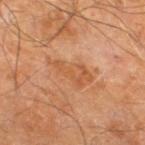Q: Was a biopsy performed?
A: no biopsy performed (imaged during a skin exam)
Q: What is the anatomic site?
A: the right leg
Q: What did automated image analysis measure?
A: an outline eccentricity of about 0.9 (0 = round, 1 = elongated) and a shape-asymmetry score of about 0.4 (0 = symmetric); a border-irregularity rating of about 5.5/10, internal color variation of about 2.5 on a 0–10 scale, and peripheral color asymmetry of about 0.5; an automated nevus-likeness rating near 0 out of 100
Q: What are the patient's age and sex?
A: male, aged 58 to 62
Q: What kind of image is this?
A: ~15 mm crop, total-body skin-cancer survey
Q: Illumination type?
A: cross-polarized